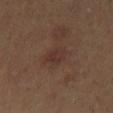The lesion is located on the leg.
A female patient aged approximately 40.
Captured under cross-polarized illumination.
A lesion tile, about 15 mm wide, cut from a 3D total-body photograph.
The lesion's longest dimension is about 3 mm.